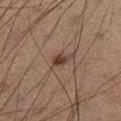| feature | finding |
|---|---|
| workup | no biopsy performed (imaged during a skin exam) |
| image-analysis metrics | a lesion area of about 3 mm² and an eccentricity of roughly 0.7; an average lesion color of about L≈38 a*≈18 b*≈24 (CIELAB), roughly 11 lightness units darker than nearby skin, and a normalized lesion–skin contrast near 9; border irregularity of about 2.5 on a 0–10 scale, a within-lesion color-variation index near 2.5/10, and a peripheral color-asymmetry measure near 1 |
| illumination | cross-polarized illumination |
| subject | male, in their mid-60s |
| diameter | ~2 mm (longest diameter) |
| image source | total-body-photography crop, ~15 mm field of view |
| site | the left lower leg |